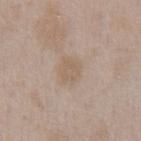Context: Measured at roughly 3 mm in maximum diameter. A 15 mm close-up extracted from a 3D total-body photography capture. A male subject aged approximately 50. The lesion is on the front of the torso.